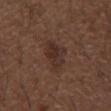follow-up: imaged on a skin check; not biopsied | anatomic site: the right forearm | imaging modality: 15 mm crop, total-body photography | subject: male, aged 48 to 52.